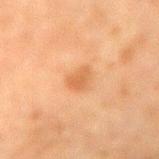  biopsy_status: not biopsied; imaged during a skin examination
  patient:
    sex: male
    age_approx: 60
  site: right upper arm
  image:
    source: total-body photography crop
    field_of_view_mm: 15
  automated_metrics:
    border_irregularity_0_10: 2.5
    color_variation_0_10: 2.5
    peripheral_color_asymmetry: 1.0